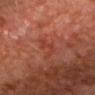• workup: total-body-photography surveillance lesion; no biopsy
• location: the head or neck
• image: ~15 mm tile from a whole-body skin photo
• patient: male, aged around 70
• lighting: cross-polarized
• size: ≈3 mm